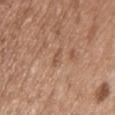The lesion was tiled from a total-body skin photograph and was not biopsied. This is a white-light tile. About 2.5 mm across. An algorithmic analysis of the crop reported a peripheral color-asymmetry measure near 0. A 15 mm crop from a total-body photograph taken for skin-cancer surveillance. The subject is a male aged approximately 50. The lesion is located on the chest.